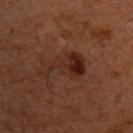Assessment:
This lesion was catalogued during total-body skin photography and was not selected for biopsy.
Acquisition and patient details:
This image is a 15 mm lesion crop taken from a total-body photograph. A male patient, about 60 years old. Automated image analysis of the tile measured an area of roughly 10 mm². The software also gave border irregularity of about 7.5 on a 0–10 scale, internal color variation of about 4.5 on a 0–10 scale, and a peripheral color-asymmetry measure near 1.5. And it measured a nevus-likeness score of about 100/100 and a lesion-detection confidence of about 100/100. Located on the chest.